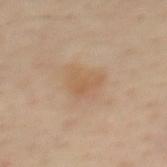workup = catalogued during a skin exam; not biopsied.